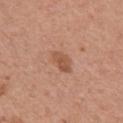Part of a total-body skin-imaging series; this lesion was reviewed on a skin check and was not flagged for biopsy. A close-up tile cropped from a whole-body skin photograph, about 15 mm across. The patient is a male roughly 45 years of age. The lesion-visualizer software estimated an area of roughly 6.5 mm² and two-axis asymmetry of about 0.15. And it measured a nevus-likeness score of about 50/100. This is a white-light tile. From the left upper arm.